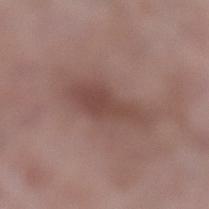<record>
  <biopsy_status>not biopsied; imaged during a skin examination</biopsy_status>
  <site>left lower leg</site>
  <lesion_size>
    <long_diameter_mm_approx>6.5</long_diameter_mm_approx>
  </lesion_size>
  <image>
    <source>total-body photography crop</source>
    <field_of_view_mm>15</field_of_view_mm>
  </image>
  <patient>
    <sex>female</sex>
    <age_approx>70</age_approx>
  </patient>
  <lighting>white-light</lighting>
  <automated_metrics>
    <eccentricity>0.9</eccentricity>
    <shape_asymmetry>0.35</shape_asymmetry>
    <cielab_L>46</cielab_L>
    <cielab_a>19</cielab_a>
    <cielab_b>22</cielab_b>
    <vs_skin_darker_L>9.0</vs_skin_darker_L>
    <vs_skin_contrast_norm>6.5</vs_skin_contrast_norm>
  </automated_metrics>
</record>